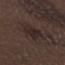The lesion was photographed on a routine skin check and not biopsied; there is no pathology result. The patient is a male aged approximately 70. Located on the left forearm. The tile uses white-light illumination. This image is a 15 mm lesion crop taken from a total-body photograph. An algorithmic analysis of the crop reported a footprint of about 9.5 mm², an eccentricity of roughly 0.9, and a symmetry-axis asymmetry near 0.35. And it measured a mean CIELAB color near L≈24 a*≈13 b*≈15, roughly 6 lightness units darker than nearby skin, and a lesion-to-skin contrast of about 7 (normalized; higher = more distinct). The software also gave a within-lesion color-variation index near 2.5/10 and peripheral color asymmetry of about 1.